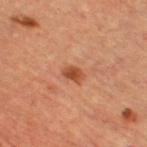<lesion>
  <image>
    <source>total-body photography crop</source>
    <field_of_view_mm>15</field_of_view_mm>
  </image>
  <lesion_size>
    <long_diameter_mm_approx>2.5</long_diameter_mm_approx>
  </lesion_size>
  <automated_metrics>
    <shape_asymmetry>0.2</shape_asymmetry>
  </automated_metrics>
  <patient>
    <sex>male</sex>
    <age_approx>60</age_approx>
  </patient>
  <site>chest</site>
  <lighting>cross-polarized</lighting>
</lesion>